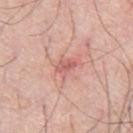biopsy status: no biopsy performed (imaged during a skin exam); subject: male, aged approximately 70; diameter: ~3 mm (longest diameter); location: the right thigh; image source: 15 mm crop, total-body photography; tile lighting: white-light illumination.